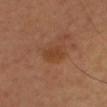Captured during whole-body skin photography for melanoma surveillance; the lesion was not biopsied.
On the head or neck.
Captured under cross-polarized illumination.
Approximately 3 mm at its widest.
Cropped from a total-body skin-imaging series; the visible field is about 15 mm.
A male patient about 55 years old.